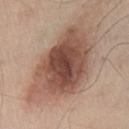* notes · no biopsy performed (imaged during a skin exam)
* patient · male, in their mid-50s
* lighting · white-light illumination
* body site · the chest
* image · ~15 mm crop, total-body skin-cancer survey